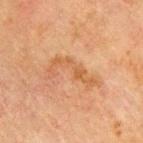• follow-up — catalogued during a skin exam; not biopsied
• tile lighting — cross-polarized illumination
• subject — male, aged 68–72
• site — the upper back
• imaging modality — 15 mm crop, total-body photography